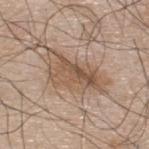follow-up: catalogued during a skin exam; not biopsied
size: about 7 mm
image: 15 mm crop, total-body photography
tile lighting: white-light
automated lesion analysis: a peripheral color-asymmetry measure near 2.5; a classifier nevus-likeness of about 60/100
subject: male, in their mid-40s
anatomic site: the upper back